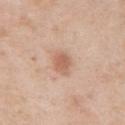No biopsy was performed on this lesion — it was imaged during a full skin examination and was not determined to be concerning.
The total-body-photography lesion software estimated an outline eccentricity of about 0.75 (0 = round, 1 = elongated) and two-axis asymmetry of about 0.2. And it measured a border-irregularity index near 2/10, internal color variation of about 2 on a 0–10 scale, and a peripheral color-asymmetry measure near 0.5. And it measured an automated nevus-likeness rating near 90 out of 100 and lesion-presence confidence of about 100/100.
A 15 mm close-up tile from a total-body photography series done for melanoma screening.
From the arm.
Imaged with white-light lighting.
A female patient aged 38–42.
About 3 mm across.